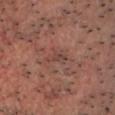Captured during whole-body skin photography for melanoma surveillance; the lesion was not biopsied.
The total-body-photography lesion software estimated about 6 CIELAB-L* units darker than the surrounding skin and a normalized border contrast of about 5.5.
Measured at roughly 3 mm in maximum diameter.
The tile uses cross-polarized illumination.
A patient aged 53 to 57.
A region of skin cropped from a whole-body photographic capture, roughly 15 mm wide.
Located on the chest.